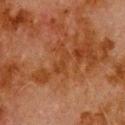<record>
<biopsy_status>not biopsied; imaged during a skin examination</biopsy_status>
</record>